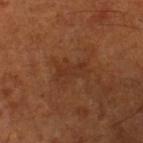{
  "biopsy_status": "not biopsied; imaged during a skin examination",
  "lesion_size": {
    "long_diameter_mm_approx": 4.0
  },
  "patient": {
    "sex": "male",
    "age_approx": 65
  },
  "site": "leg",
  "image": {
    "source": "total-body photography crop",
    "field_of_view_mm": 15
  },
  "lighting": "cross-polarized"
}